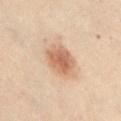Imaged during a routine full-body skin examination; the lesion was not biopsied and no histopathology is available.
A female subject aged 28–32.
From the abdomen.
A 15 mm close-up tile from a total-body photography series done for melanoma screening.
The total-body-photography lesion software estimated an area of roughly 11 mm², an eccentricity of roughly 0.6, and two-axis asymmetry of about 0.15. The analysis additionally found a lesion color around L≈50 a*≈17 b*≈27 in CIELAB, about 10 CIELAB-L* units darker than the surrounding skin, and a lesion-to-skin contrast of about 7.5 (normalized; higher = more distinct). The analysis additionally found a classifier nevus-likeness of about 100/100 and lesion-presence confidence of about 100/100.